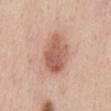notes = total-body-photography surveillance lesion; no biopsy
image = total-body-photography crop, ~15 mm field of view
subject = female, in their mid-40s
anatomic site = the abdomen